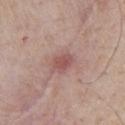Notes:
• subject — male, aged 73 to 77
• imaging modality — ~15 mm crop, total-body skin-cancer survey
• site — the front of the torso